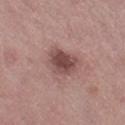Recorded during total-body skin imaging; not selected for excision or biopsy.
The tile uses white-light illumination.
Longest diameter approximately 4 mm.
A female patient in their mid-60s.
The lesion is on the leg.
A 15 mm close-up extracted from a 3D total-body photography capture.
An algorithmic analysis of the crop reported an area of roughly 9 mm² and a shape-asymmetry score of about 0.25 (0 = symmetric). And it measured a mean CIELAB color near L≈47 a*≈21 b*≈20, roughly 12 lightness units darker than nearby skin, and a lesion-to-skin contrast of about 9 (normalized; higher = more distinct). It also reported a classifier nevus-likeness of about 65/100 and a detector confidence of about 100 out of 100 that the crop contains a lesion.